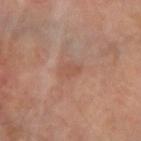Imaged during a routine full-body skin examination; the lesion was not biopsied and no histopathology is available.
Automated tile analysis of the lesion measured a border-irregularity index near 2.5/10, a color-variation rating of about 1.5/10, and a peripheral color-asymmetry measure near 0.5.
A roughly 15 mm field-of-view crop from a total-body skin photograph.
The patient is a female approximately 65 years of age.
The lesion's longest dimension is about 2.5 mm.
On the right forearm.